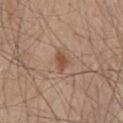biopsy status = imaged on a skin check; not biopsied | anatomic site = the lower back | image-analysis metrics = an area of roughly 3.5 mm², an eccentricity of roughly 0.75, and two-axis asymmetry of about 0.35 | patient = male, about 45 years old | size = ~2.5 mm (longest diameter) | imaging modality = total-body-photography crop, ~15 mm field of view.